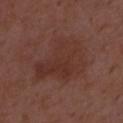notes=no biopsy performed (imaged during a skin exam); lighting=white-light; image=~15 mm crop, total-body skin-cancer survey; size=≈7.5 mm; patient=male, approximately 50 years of age.